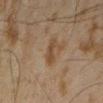{"biopsy_status": "not biopsied; imaged during a skin examination", "patient": {"sex": "male", "age_approx": 45}, "lesion_size": {"long_diameter_mm_approx": 2.5}, "image": {"source": "total-body photography crop", "field_of_view_mm": 15}, "automated_metrics": {"border_irregularity_0_10": 3.0, "peripheral_color_asymmetry": 0.5}, "lighting": "cross-polarized", "site": "left forearm"}